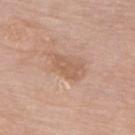Measured at roughly 3.5 mm in maximum diameter. Located on the upper back. A female subject aged 68–72. Automated tile analysis of the lesion measured a shape-asymmetry score of about 0.3 (0 = symmetric). And it measured a lesion–skin lightness drop of about 8 and a normalized lesion–skin contrast near 5.5. The software also gave a nevus-likeness score of about 0/100. Cropped from a whole-body photographic skin survey; the tile spans about 15 mm.